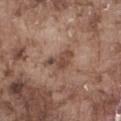Assessment:
Recorded during total-body skin imaging; not selected for excision or biopsy.
Acquisition and patient details:
This image is a 15 mm lesion crop taken from a total-body photograph. From the right thigh. The tile uses white-light illumination. The subject is a male roughly 75 years of age.